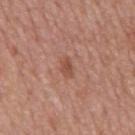| feature | finding |
|---|---|
| follow-up | total-body-photography surveillance lesion; no biopsy |
| automated lesion analysis | border irregularity of about 3 on a 0–10 scale, a color-variation rating of about 1/10, and a peripheral color-asymmetry measure near 0.5; a nevus-likeness score of about 0/100 |
| diameter | ≈2.5 mm |
| location | the back |
| image | ~15 mm crop, total-body skin-cancer survey |
| illumination | white-light |
| subject | male, in their mid- to late 60s |